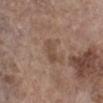No biopsy was performed on this lesion — it was imaged during a full skin examination and was not determined to be concerning.
From the left lower leg.
About 3 mm across.
Automated image analysis of the tile measured a color-variation rating of about 2/10 and a peripheral color-asymmetry measure near 0.5. The software also gave a nevus-likeness score of about 0/100.
A roughly 15 mm field-of-view crop from a total-body skin photograph.
The patient is a female in their mid- to late 80s or older.